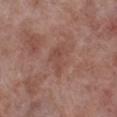Assessment: Part of a total-body skin-imaging series; this lesion was reviewed on a skin check and was not flagged for biopsy.